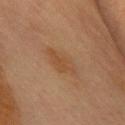Assessment:
Part of a total-body skin-imaging series; this lesion was reviewed on a skin check and was not flagged for biopsy.
Acquisition and patient details:
Measured at roughly 4.5 mm in maximum diameter. The total-body-photography lesion software estimated border irregularity of about 3 on a 0–10 scale, internal color variation of about 2 on a 0–10 scale, and peripheral color asymmetry of about 0.5. The lesion is located on the chest. The subject is a female approximately 60 years of age. Captured under cross-polarized illumination. A lesion tile, about 15 mm wide, cut from a 3D total-body photograph.